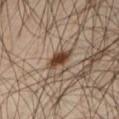notes: total-body-photography surveillance lesion; no biopsy | image: 15 mm crop, total-body photography | location: the right thigh | patient: male, aged around 45.